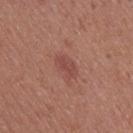Clinical impression: The lesion was tiled from a total-body skin photograph and was not biopsied.